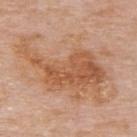Case summary:
– follow-up: no biopsy performed (imaged during a skin exam)
– image: ~15 mm crop, total-body skin-cancer survey
– site: the upper back
– image-analysis metrics: a lesion area of about 65 mm², an eccentricity of roughly 0.8, and a symmetry-axis asymmetry near 0.3; a mean CIELAB color near L≈60 a*≈21 b*≈34, a lesion–skin lightness drop of about 8, and a lesion-to-skin contrast of about 6 (normalized; higher = more distinct)
– diameter: ~12.5 mm (longest diameter)
– patient: male, aged 73–77